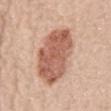follow-up = no biopsy performed (imaged during a skin exam); patient = female, aged 68–72; location = the front of the torso; image source = ~15 mm crop, total-body skin-cancer survey; tile lighting = white-light.